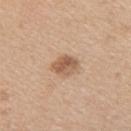Assessment: Part of a total-body skin-imaging series; this lesion was reviewed on a skin check and was not flagged for biopsy. Background: This is a white-light tile. A lesion tile, about 15 mm wide, cut from a 3D total-body photograph. The patient is a female roughly 40 years of age. Longest diameter approximately 3 mm. Located on the upper back.